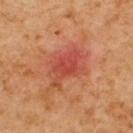No biopsy was performed on this lesion — it was imaged during a full skin examination and was not determined to be concerning. This image is a 15 mm lesion crop taken from a total-body photograph. The lesion's longest dimension is about 5 mm. On the upper back. Imaged with cross-polarized lighting. A male subject, aged 58–62.